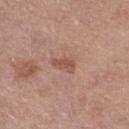A lesion tile, about 15 mm wide, cut from a 3D total-body photograph. The patient is a female in their mid-50s. The recorded lesion diameter is about 3 mm. On the right thigh. The total-body-photography lesion software estimated an area of roughly 3.5 mm². The tile uses white-light illumination.